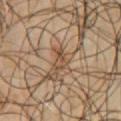Notes:
- notes · imaged on a skin check; not biopsied
- tile lighting · cross-polarized illumination
- location · the chest
- imaging modality · total-body-photography crop, ~15 mm field of view
- subject · male, in their mid- to late 60s